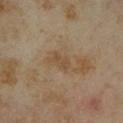Captured during whole-body skin photography for melanoma surveillance; the lesion was not biopsied. The subject is a female aged 33–37. Captured under cross-polarized illumination. The lesion is located on the right forearm. The recorded lesion diameter is about 2.5 mm. A 15 mm close-up extracted from a 3D total-body photography capture. Automated image analysis of the tile measured a lesion color around L≈46 a*≈14 b*≈31 in CIELAB, a lesion–skin lightness drop of about 6, and a normalized border contrast of about 5.5. It also reported a border-irregularity rating of about 3/10, a color-variation rating of about 1/10, and radial color variation of about 0.5.